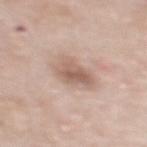follow-up: catalogued during a skin exam; not biopsied
lesion diameter: about 5 mm
subject: female, roughly 50 years of age
location: the upper back
automated lesion analysis: a footprint of about 12 mm² and two-axis asymmetry of about 0.2; a mean CIELAB color near L≈61 a*≈17 b*≈26, a lesion–skin lightness drop of about 10, and a normalized lesion–skin contrast near 6.5; border irregularity of about 2.5 on a 0–10 scale, internal color variation of about 5 on a 0–10 scale, and peripheral color asymmetry of about 2; a lesion-detection confidence of about 100/100
acquisition: 15 mm crop, total-body photography
lighting: white-light